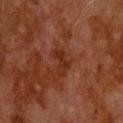Impression:
Recorded during total-body skin imaging; not selected for excision or biopsy.
Background:
Longest diameter approximately 3 mm. The lesion is located on the back. A 15 mm close-up tile from a total-body photography series done for melanoma screening. Captured under cross-polarized illumination. The patient is a male aged approximately 80. Automated tile analysis of the lesion measured a nevus-likeness score of about 0/100 and lesion-presence confidence of about 100/100.